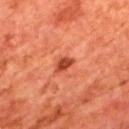Assessment: No biopsy was performed on this lesion — it was imaged during a full skin examination and was not determined to be concerning. Clinical summary: A male subject, in their mid- to late 60s. A lesion tile, about 15 mm wide, cut from a 3D total-body photograph. Located on the upper back.